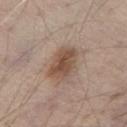<lesion>
<biopsy_status>not biopsied; imaged during a skin examination</biopsy_status>
<image>
  <source>total-body photography crop</source>
  <field_of_view_mm>15</field_of_view_mm>
</image>
<lighting>white-light</lighting>
<lesion_size>
  <long_diameter_mm_approx>4.5</long_diameter_mm_approx>
</lesion_size>
<patient>
  <sex>male</sex>
  <age_approx>70</age_approx>
</patient>
<site>chest</site>
</lesion>